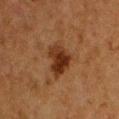{"biopsy_status": "not biopsied; imaged during a skin examination", "lesion_size": {"long_diameter_mm_approx": 4.5}, "image": {"source": "total-body photography crop", "field_of_view_mm": 15}, "patient": {"sex": "female", "age_approx": 50}, "automated_metrics": {"cielab_L": 27, "cielab_a": 20, "cielab_b": 28, "vs_skin_darker_L": 10.0, "vs_skin_contrast_norm": 10.5, "border_irregularity_0_10": 3.0, "color_variation_0_10": 4.5}, "lighting": "cross-polarized", "site": "front of the torso"}